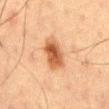The lesion was photographed on a routine skin check and not biopsied; there is no pathology result. The lesion is located on the mid back. A male patient, aged around 60. This image is a 15 mm lesion crop taken from a total-body photograph. Captured under cross-polarized illumination.